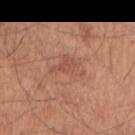Assessment: Recorded during total-body skin imaging; not selected for excision or biopsy. Acquisition and patient details: The lesion-visualizer software estimated an average lesion color of about L≈52 a*≈23 b*≈31 (CIELAB) and a lesion–skin lightness drop of about 7. The analysis additionally found a border-irregularity index near 6.5/10, a color-variation rating of about 2/10, and peripheral color asymmetry of about 0.5. The analysis additionally found an automated nevus-likeness rating near 0 out of 100 and a lesion-detection confidence of about 100/100. The recorded lesion diameter is about 4 mm. A roughly 15 mm field-of-view crop from a total-body skin photograph. The tile uses white-light illumination. The patient is a male roughly 70 years of age. From the right upper arm.